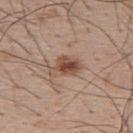No biopsy was performed on this lesion — it was imaged during a full skin examination and was not determined to be concerning.
The lesion is on the upper back.
Automated image analysis of the tile measured two-axis asymmetry of about 0.35. And it measured a mean CIELAB color near L≈49 a*≈19 b*≈28. The analysis additionally found a border-irregularity index near 3.5/10 and radial color variation of about 2.
A close-up tile cropped from a whole-body skin photograph, about 15 mm across.
Approximately 4 mm at its widest.
Imaged with white-light lighting.
The subject is a male roughly 65 years of age.